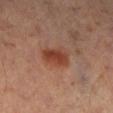Q: What is the imaging modality?
A: ~15 mm crop, total-body skin-cancer survey
Q: Who is the patient?
A: male, aged approximately 55
Q: Lesion location?
A: the leg